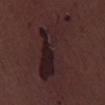| field | value |
|---|---|
| follow-up | catalogued during a skin exam; not biopsied |
| patient | male, aged approximately 30 |
| imaging modality | 15 mm crop, total-body photography |
| body site | the right lower leg |